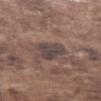The lesion was tiled from a total-body skin photograph and was not biopsied. Located on the left forearm. A male subject, aged 73 to 77. A roughly 15 mm field-of-view crop from a total-body skin photograph.